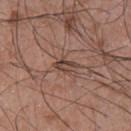workup: total-body-photography surveillance lesion; no biopsy | anatomic site: the upper back | lighting: white-light illumination | image: ~15 mm crop, total-body skin-cancer survey | automated lesion analysis: a lesion area of about 3 mm² and a shape eccentricity near 0.85; a lesion color around L≈42 a*≈18 b*≈24 in CIELAB, roughly 10 lightness units darker than nearby skin, and a normalized lesion–skin contrast near 8; internal color variation of about 2.5 on a 0–10 scale; a nevus-likeness score of about 0/100 and a lesion-detection confidence of about 55/100 | patient: male, roughly 50 years of age | lesion size: ≈3 mm.